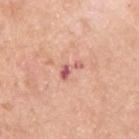<record>
<biopsy_status>not biopsied; imaged during a skin examination</biopsy_status>
<site>upper back</site>
<patient>
  <sex>male</sex>
  <age_approx>70</age_approx>
</patient>
<automated_metrics>
  <area_mm2_approx>3.0</area_mm2_approx>
  <eccentricity>0.9</eccentricity>
  <shape_asymmetry>0.7</shape_asymmetry>
  <cielab_L>60</cielab_L>
  <cielab_a>29</cielab_a>
  <cielab_b>26</cielab_b>
  <vs_skin_darker_L>12.0</vs_skin_darker_L>
  <vs_skin_contrast_norm>8.0</vs_skin_contrast_norm>
  <border_irregularity_0_10>7.0</border_irregularity_0_10>
  <color_variation_0_10>2.0</color_variation_0_10>
  <peripheral_color_asymmetry>0.5</peripheral_color_asymmetry>
  <nevus_likeness_0_100>0</nevus_likeness_0_100>
  <lesion_detection_confidence_0_100>100</lesion_detection_confidence_0_100>
</automated_metrics>
<image>
  <source>total-body photography crop</source>
  <field_of_view_mm>15</field_of_view_mm>
</image>
<lesion_size>
  <long_diameter_mm_approx>3.0</long_diameter_mm_approx>
</lesion_size>
<lighting>white-light</lighting>
</record>